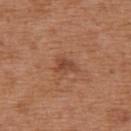Imaged during a routine full-body skin examination; the lesion was not biopsied and no histopathology is available.
A 15 mm crop from a total-body photograph taken for skin-cancer surveillance.
A female subject aged approximately 40.
The tile uses white-light illumination.
The lesion-visualizer software estimated a footprint of about 3 mm², an outline eccentricity of about 0.8 (0 = round, 1 = elongated), and a symmetry-axis asymmetry near 0.45. The software also gave a border-irregularity index near 4.5/10.
On the upper back.
About 2.5 mm across.